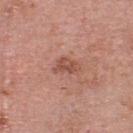This lesion was catalogued during total-body skin photography and was not selected for biopsy. The lesion is located on the head or neck. Cropped from a whole-body photographic skin survey; the tile spans about 15 mm. A female patient, in their 60s. The recorded lesion diameter is about 3 mm. The tile uses white-light illumination.